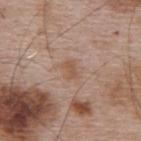  biopsy_status: not biopsied; imaged during a skin examination
  image:
    source: total-body photography crop
    field_of_view_mm: 15
  site: upper back
  patient:
    sex: male
    age_approx: 65